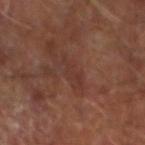An algorithmic analysis of the crop reported a lesion color around L≈32 a*≈20 b*≈23 in CIELAB, roughly 5 lightness units darker than nearby skin, and a normalized border contrast of about 5. From the right forearm. Captured under cross-polarized illumination. Measured at roughly 4 mm in maximum diameter. A 15 mm close-up extracted from a 3D total-body photography capture. A male patient, approximately 65 years of age.